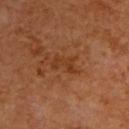Part of a total-body skin-imaging series; this lesion was reviewed on a skin check and was not flagged for biopsy. The tile uses cross-polarized illumination. The patient is a male about 60 years old. Located on the upper back. An algorithmic analysis of the crop reported a lesion area of about 3.5 mm², an outline eccentricity of about 0.85 (0 = round, 1 = elongated), and a shape-asymmetry score of about 0.55 (0 = symmetric). A close-up tile cropped from a whole-body skin photograph, about 15 mm across. Measured at roughly 3 mm in maximum diameter.